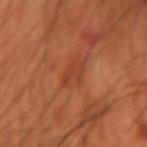Captured during whole-body skin photography for melanoma surveillance; the lesion was not biopsied.
A close-up tile cropped from a whole-body skin photograph, about 15 mm across.
The lesion is on the arm.
Imaged with cross-polarized lighting.
A male subject, about 70 years old.